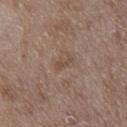Q: Was this lesion biopsied?
A: total-body-photography surveillance lesion; no biopsy
Q: How was the tile lit?
A: white-light
Q: How large is the lesion?
A: ~2.5 mm (longest diameter)
Q: What is the imaging modality?
A: total-body-photography crop, ~15 mm field of view
Q: Who is the patient?
A: male, roughly 50 years of age
Q: Automated lesion metrics?
A: a border-irregularity index near 6/10, a color-variation rating of about 0/10, and peripheral color asymmetry of about 0; a nevus-likeness score of about 0/100 and a detector confidence of about 100 out of 100 that the crop contains a lesion
Q: What is the anatomic site?
A: the right upper arm